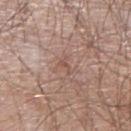Recorded during total-body skin imaging; not selected for excision or biopsy. The patient is a male aged 63 to 67. This image is a 15 mm lesion crop taken from a total-body photograph. Imaged with white-light lighting. Approximately 2.5 mm at its widest. From the right upper arm. An algorithmic analysis of the crop reported an outline eccentricity of about 0.85 (0 = round, 1 = elongated). The software also gave a lesion color around L≈52 a*≈19 b*≈24 in CIELAB and a lesion-to-skin contrast of about 5 (normalized; higher = more distinct).